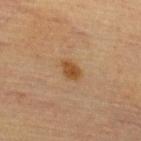Case summary:
- biopsy status · catalogued during a skin exam; not biopsied
- tile lighting · cross-polarized illumination
- anatomic site · the upper back
- image · ~15 mm tile from a whole-body skin photo
- lesion size · ~2.5 mm (longest diameter)
- patient · female, aged 63–67